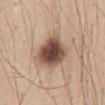Recorded during total-body skin imaging; not selected for excision or biopsy.
A male subject, roughly 45 years of age.
The recorded lesion diameter is about 5 mm.
A roughly 15 mm field-of-view crop from a total-body skin photograph.
On the front of the torso.
Captured under white-light illumination.